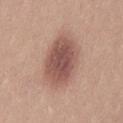Cropped from a whole-body photographic skin survey; the tile spans about 15 mm. A female subject aged around 20. The lesion's longest dimension is about 7 mm. On the left thigh. The tile uses white-light illumination.